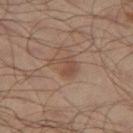The lesion was tiled from a total-body skin photograph and was not biopsied.
About 3 mm across.
Automated tile analysis of the lesion measured an area of roughly 4.5 mm², an eccentricity of roughly 0.75, and a symmetry-axis asymmetry near 0.55. And it measured a border-irregularity rating of about 6/10, a color-variation rating of about 1.5/10, and a peripheral color-asymmetry measure near 0.5. And it measured a nevus-likeness score of about 10/100.
A male subject, aged approximately 65.
Captured under cross-polarized illumination.
A close-up tile cropped from a whole-body skin photograph, about 15 mm across.
Located on the left thigh.